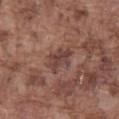This lesion was catalogued during total-body skin photography and was not selected for biopsy. A close-up tile cropped from a whole-body skin photograph, about 15 mm across. Automated tile analysis of the lesion measured a border-irregularity rating of about 3.5/10. It also reported a nevus-likeness score of about 0/100. About 4 mm across. On the front of the torso. The subject is a male aged approximately 75.